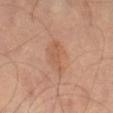biopsy status = total-body-photography surveillance lesion; no biopsy | image-analysis metrics = a footprint of about 9.5 mm², an eccentricity of roughly 0.85, and a symmetry-axis asymmetry near 0.3; a mean CIELAB color near L≈54 a*≈20 b*≈31, a lesion–skin lightness drop of about 6, and a normalized border contrast of about 5; lesion-presence confidence of about 100/100 | acquisition = ~15 mm crop, total-body skin-cancer survey | subject = male, aged around 60 | lesion diameter = about 5 mm | site = the right leg.